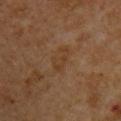Captured during whole-body skin photography for melanoma surveillance; the lesion was not biopsied. The lesion is located on the left upper arm. This image is a 15 mm lesion crop taken from a total-body photograph. A male subject, aged 58–62.